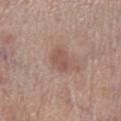Impression:
Imaged during a routine full-body skin examination; the lesion was not biopsied and no histopathology is available.
Context:
The tile uses white-light illumination. Cropped from a total-body skin-imaging series; the visible field is about 15 mm. The recorded lesion diameter is about 3 mm. A male subject, aged 68 to 72. On the left lower leg.